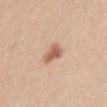Background:
A female subject, about 25 years old. From the abdomen. A close-up tile cropped from a whole-body skin photograph, about 15 mm across.